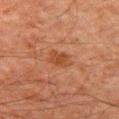Clinical impression:
The lesion was photographed on a routine skin check and not biopsied; there is no pathology result.
Context:
The lesion is located on the chest. This image is a 15 mm lesion crop taken from a total-body photograph. A male patient, aged 58 to 62. The tile uses cross-polarized illumination. About 3 mm across. The lesion-visualizer software estimated a border-irregularity index near 2.5/10, internal color variation of about 1.5 on a 0–10 scale, and a peripheral color-asymmetry measure near 0.5.